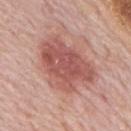| field | value |
|---|---|
| notes | no biopsy performed (imaged during a skin exam) |
| automated lesion analysis | a shape eccentricity near 0.7 and a shape-asymmetry score of about 0.25 (0 = symmetric); border irregularity of about 3.5 on a 0–10 scale and radial color variation of about 1.5; a classifier nevus-likeness of about 60/100 and a detector confidence of about 100 out of 100 that the crop contains a lesion |
| site | the mid back |
| lighting | white-light illumination |
| size | about 7.5 mm |
| subject | male, approximately 75 years of age |
| acquisition | ~15 mm crop, total-body skin-cancer survey |